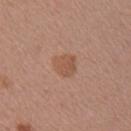workup=catalogued during a skin exam; not biopsied | subject=female, in their mid-30s | tile lighting=white-light illumination | lesion size=≈2.5 mm | image=~15 mm tile from a whole-body skin photo | anatomic site=the arm.